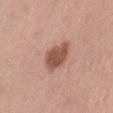| field | value |
|---|---|
| notes | total-body-photography surveillance lesion; no biopsy |
| TBP lesion metrics | a footprint of about 9 mm², a shape eccentricity near 0.75, and two-axis asymmetry of about 0.2; roughly 13 lightness units darker than nearby skin and a lesion-to-skin contrast of about 9 (normalized; higher = more distinct); a border-irregularity rating of about 2.5/10, a color-variation rating of about 3/10, and peripheral color asymmetry of about 1; lesion-presence confidence of about 100/100 |
| patient | male, roughly 45 years of age |
| site | the back |
| lighting | white-light illumination |
| imaging modality | ~15 mm tile from a whole-body skin photo |
| lesion size | about 4 mm |